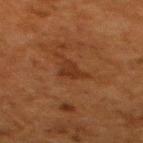Recorded during total-body skin imaging; not selected for excision or biopsy. This is a cross-polarized tile. A 15 mm crop from a total-body photograph taken for skin-cancer surveillance. The lesion is on the upper back. Measured at roughly 3 mm in maximum diameter. A female subject in their 50s.